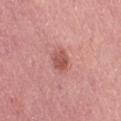Assessment:
This lesion was catalogued during total-body skin photography and was not selected for biopsy.
Context:
A female patient, aged 53–57. A 15 mm close-up tile from a total-body photography series done for melanoma screening. The lesion is located on the front of the torso.